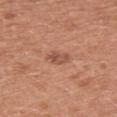Imaged during a routine full-body skin examination; the lesion was not biopsied and no histopathology is available. Longest diameter approximately 2.5 mm. A close-up tile cropped from a whole-body skin photograph, about 15 mm across. A female patient about 40 years old. Located on the arm. An algorithmic analysis of the crop reported an automated nevus-likeness rating near 5 out of 100 and a lesion-detection confidence of about 100/100.